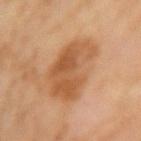Clinical summary:
A female subject, aged 58 to 62. A 15 mm crop from a total-body photograph taken for skin-cancer surveillance. An algorithmic analysis of the crop reported a mean CIELAB color near L≈50 a*≈21 b*≈35, roughly 9 lightness units darker than nearby skin, and a normalized border contrast of about 7. The analysis additionally found a border-irregularity index near 4/10, a within-lesion color-variation index near 4.5/10, and a peripheral color-asymmetry measure near 1.5. The analysis additionally found a nevus-likeness score of about 25/100 and a detector confidence of about 100 out of 100 that the crop contains a lesion. From the left upper arm. This is a cross-polarized tile. Approximately 6 mm at its widest.